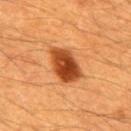biopsy status: total-body-photography surveillance lesion; no biopsy
tile lighting: cross-polarized
subject: male, aged approximately 60
TBP lesion metrics: a lesion color around L≈44 a*≈30 b*≈41 in CIELAB and roughly 17 lightness units darker than nearby skin; a border-irregularity index near 2/10, internal color variation of about 5.5 on a 0–10 scale, and peripheral color asymmetry of about 2; a classifier nevus-likeness of about 100/100 and a lesion-detection confidence of about 100/100
size: ~5 mm (longest diameter)
imaging modality: 15 mm crop, total-body photography
body site: the back